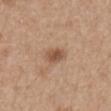follow-up=no biopsy performed (imaged during a skin exam) | imaging modality=15 mm crop, total-body photography | tile lighting=white-light illumination | subject=male, roughly 65 years of age | anatomic site=the right upper arm | lesion diameter=≈3 mm | automated lesion analysis=an area of roughly 5 mm², a shape eccentricity near 0.7, and a symmetry-axis asymmetry near 0.2; an average lesion color of about L≈53 a*≈19 b*≈31 (CIELAB), about 10 CIELAB-L* units darker than the surrounding skin, and a normalized lesion–skin contrast near 7.5; border irregularity of about 1.5 on a 0–10 scale, a color-variation rating of about 3.5/10, and peripheral color asymmetry of about 1.